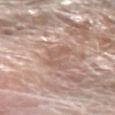No biopsy was performed on this lesion — it was imaged during a full skin examination and was not determined to be concerning.
Approximately 4.5 mm at its widest.
This is a white-light tile.
A 15 mm close-up extracted from a 3D total-body photography capture.
The total-body-photography lesion software estimated an area of roughly 6 mm², an eccentricity of roughly 0.9, and two-axis asymmetry of about 0.6. The software also gave an average lesion color of about L≈58 a*≈19 b*≈25 (CIELAB) and a lesion–skin lightness drop of about 7.
The subject is a male aged around 80.
From the arm.